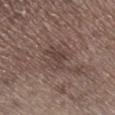notes = imaged on a skin check; not biopsied
image source = ~15 mm crop, total-body skin-cancer survey
patient = male, about 70 years old
location = the right lower leg